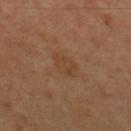Recorded during total-body skin imaging; not selected for excision or biopsy. The subject is a male roughly 50 years of age. This image is a 15 mm lesion crop taken from a total-body photograph. Longest diameter approximately 3.5 mm. Captured under cross-polarized illumination. Automated image analysis of the tile measured a footprint of about 6 mm² and two-axis asymmetry of about 0.2. The software also gave a border-irregularity index near 2.5/10 and radial color variation of about 0.5. From the upper back.